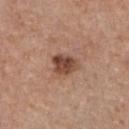Assessment:
The lesion was tiled from a total-body skin photograph and was not biopsied.
Clinical summary:
On the chest. A female subject, aged around 35. The total-body-photography lesion software estimated a within-lesion color-variation index near 4.5/10 and peripheral color asymmetry of about 2. The software also gave a detector confidence of about 100 out of 100 that the crop contains a lesion. A 15 mm crop from a total-body photograph taken for skin-cancer surveillance.